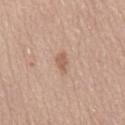Q: Was this lesion biopsied?
A: no biopsy performed (imaged during a skin exam)
Q: What is the anatomic site?
A: the mid back
Q: Who is the patient?
A: female, approximately 50 years of age
Q: What kind of image is this?
A: ~15 mm tile from a whole-body skin photo